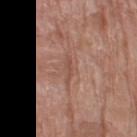Clinical impression:
Part of a total-body skin-imaging series; this lesion was reviewed on a skin check and was not flagged for biopsy.
Context:
Captured under white-light illumination. A lesion tile, about 15 mm wide, cut from a 3D total-body photograph. The subject is a female approximately 75 years of age. Located on the left thigh. Automated image analysis of the tile measured a mean CIELAB color near L≈50 a*≈22 b*≈27 and a normalized lesion–skin contrast near 5.5. Approximately 3 mm at its widest.